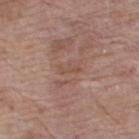Clinical impression:
The lesion was tiled from a total-body skin photograph and was not biopsied.
Acquisition and patient details:
A female subject, aged 68 to 72. An algorithmic analysis of the crop reported an average lesion color of about L≈50 a*≈19 b*≈27 (CIELAB), roughly 5 lightness units darker than nearby skin, and a normalized border contrast of about 4.5. And it measured border irregularity of about 6.5 on a 0–10 scale, a color-variation rating of about 0/10, and peripheral color asymmetry of about 0. It also reported a classifier nevus-likeness of about 0/100 and lesion-presence confidence of about 85/100. The lesion is located on the right thigh. The lesion's longest dimension is about 2.5 mm. A 15 mm crop from a total-body photograph taken for skin-cancer surveillance. Captured under white-light illumination.